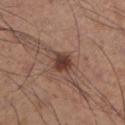Recorded during total-body skin imaging; not selected for excision or biopsy. From the leg. A 15 mm close-up tile from a total-body photography series done for melanoma screening. The subject is a male aged approximately 35.